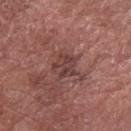– biopsy status · catalogued during a skin exam; not biopsied
– tile lighting · white-light
– automated lesion analysis · a border-irregularity rating of about 4/10, a color-variation rating of about 3/10, and a peripheral color-asymmetry measure near 1
– location · the left forearm
– lesion size · ~3 mm (longest diameter)
– subject · male, roughly 75 years of age
– image source · ~15 mm crop, total-body skin-cancer survey